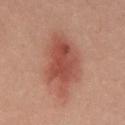Q: Was this lesion biopsied?
A: total-body-photography surveillance lesion; no biopsy
Q: Lesion location?
A: the abdomen
Q: How was the tile lit?
A: cross-polarized illumination
Q: How large is the lesion?
A: about 7 mm
Q: How was this image acquired?
A: total-body-photography crop, ~15 mm field of view
Q: Patient demographics?
A: male, aged 38–42
Q: Automated lesion metrics?
A: a lesion color around L≈38 a*≈22 b*≈23 in CIELAB, about 9 CIELAB-L* units darker than the surrounding skin, and a normalized border contrast of about 8; a border-irregularity index near 4/10, internal color variation of about 4 on a 0–10 scale, and a peripheral color-asymmetry measure near 1; a classifier nevus-likeness of about 95/100 and lesion-presence confidence of about 100/100